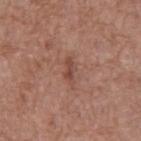<tbp_lesion>
<biopsy_status>not biopsied; imaged during a skin examination</biopsy_status>
<lighting>white-light</lighting>
<patient>
  <sex>male</sex>
  <age_approx>75</age_approx>
</patient>
<image>
  <source>total-body photography crop</source>
  <field_of_view_mm>15</field_of_view_mm>
</image>
<lesion_size>
  <long_diameter_mm_approx>3.0</long_diameter_mm_approx>
</lesion_size>
<site>front of the torso</site>
</tbp_lesion>